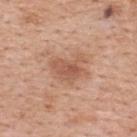The lesion was tiled from a total-body skin photograph and was not biopsied.
The lesion is on the upper back.
The patient is a female in their 40s.
The total-body-photography lesion software estimated a lesion area of about 9 mm², an eccentricity of roughly 0.7, and a shape-asymmetry score of about 0.4 (0 = symmetric). The software also gave a mean CIELAB color near L≈56 a*≈23 b*≈31, roughly 9 lightness units darker than nearby skin, and a normalized lesion–skin contrast near 6.5. And it measured border irregularity of about 4.5 on a 0–10 scale, internal color variation of about 2.5 on a 0–10 scale, and radial color variation of about 1. It also reported an automated nevus-likeness rating near 25 out of 100 and a detector confidence of about 100 out of 100 that the crop contains a lesion.
A region of skin cropped from a whole-body photographic capture, roughly 15 mm wide.